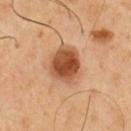Impression:
Part of a total-body skin-imaging series; this lesion was reviewed on a skin check and was not flagged for biopsy.
Clinical summary:
A male patient aged 48 to 52. A 15 mm crop from a total-body photograph taken for skin-cancer surveillance. The lesion is on the chest.